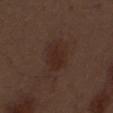Findings:
• biopsy status: total-body-photography surveillance lesion; no biopsy
• lesion diameter: ≈4 mm
• subject: male, in their 70s
• image source: total-body-photography crop, ~15 mm field of view
• illumination: white-light illumination
• image-analysis metrics: an average lesion color of about L≈26 a*≈17 b*≈22 (CIELAB), about 5 CIELAB-L* units darker than the surrounding skin, and a lesion-to-skin contrast of about 6 (normalized; higher = more distinct)
• location: the abdomen